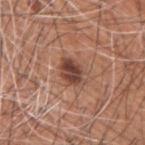Assessment: The lesion was photographed on a routine skin check and not biopsied; there is no pathology result. Acquisition and patient details: Located on the right upper arm. A roughly 15 mm field-of-view crop from a total-body skin photograph. The lesion's longest dimension is about 4 mm. A male subject, roughly 60 years of age.